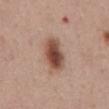No biopsy was performed on this lesion — it was imaged during a full skin examination and was not determined to be concerning.
A 15 mm close-up tile from a total-body photography series done for melanoma screening.
On the abdomen.
A male patient, aged 48 to 52.
The lesion-visualizer software estimated a footprint of about 11 mm² and two-axis asymmetry of about 0.15. The software also gave an automated nevus-likeness rating near 100 out of 100.